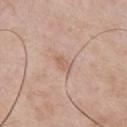Clinical impression:
Part of a total-body skin-imaging series; this lesion was reviewed on a skin check and was not flagged for biopsy.
Background:
Measured at roughly 2.5 mm in maximum diameter. A male patient about 55 years old. Cropped from a total-body skin-imaging series; the visible field is about 15 mm. Imaged with white-light lighting. The lesion-visualizer software estimated a normalized border contrast of about 5.5. From the chest.